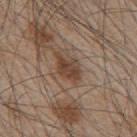Imaged during a routine full-body skin examination; the lesion was not biopsied and no histopathology is available. Cropped from a whole-body photographic skin survey; the tile spans about 15 mm. The total-body-photography lesion software estimated an area of roughly 6 mm² and a shape eccentricity near 0.8. The analysis additionally found about 10 CIELAB-L* units darker than the surrounding skin and a normalized border contrast of about 8.5. The software also gave an automated nevus-likeness rating near 60 out of 100 and a lesion-detection confidence of about 100/100. Approximately 3.5 mm at its widest. From the mid back. The patient is a male aged 43–47.